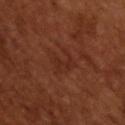The lesion was tiled from a total-body skin photograph and was not biopsied.
This image is a 15 mm lesion crop taken from a total-body photograph.
The patient is a male aged approximately 65.
The tile uses cross-polarized illumination.
Measured at roughly 3.5 mm in maximum diameter.